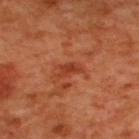The subject is a male approximately 50 years of age.
Longest diameter approximately 3 mm.
Captured under cross-polarized illumination.
An algorithmic analysis of the crop reported a within-lesion color-variation index near 1.5/10 and peripheral color asymmetry of about 0.5. And it measured a nevus-likeness score of about 0/100 and a lesion-detection confidence of about 100/100.
Located on the upper back.
A 15 mm close-up extracted from a 3D total-body photography capture.